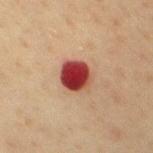Clinical impression: Part of a total-body skin-imaging series; this lesion was reviewed on a skin check and was not flagged for biopsy. Background: The lesion's longest dimension is about 4 mm. The tile uses cross-polarized illumination. Located on the chest. Cropped from a total-body skin-imaging series; the visible field is about 15 mm. A male patient aged 58 to 62.